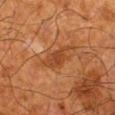{
  "biopsy_status": "not biopsied; imaged during a skin examination",
  "patient": {
    "sex": "male",
    "age_approx": 80
  },
  "site": "left lower leg",
  "image": {
    "source": "total-body photography crop",
    "field_of_view_mm": 15
  }
}